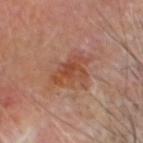follow-up = imaged on a skin check; not biopsied
imaging modality = ~15 mm tile from a whole-body skin photo
patient = male, aged approximately 60
size = ≈4 mm
anatomic site = the head or neck
illumination = cross-polarized illumination